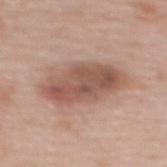{"biopsy_status": "not biopsied; imaged during a skin examination", "image": {"source": "total-body photography crop", "field_of_view_mm": 15}, "lesion_size": {"long_diameter_mm_approx": 8.0}, "patient": {"sex": "female", "age_approx": 60}, "site": "back"}